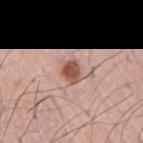Part of a total-body skin-imaging series; this lesion was reviewed on a skin check and was not flagged for biopsy.
A male subject aged 53 to 57.
The lesion-visualizer software estimated a border-irregularity index near 2.5/10, a color-variation rating of about 4/10, and radial color variation of about 1. It also reported a classifier nevus-likeness of about 100/100 and a lesion-detection confidence of about 100/100.
The tile uses white-light illumination.
A roughly 15 mm field-of-view crop from a total-body skin photograph.